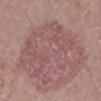biopsy status: catalogued during a skin exam; not biopsied | body site: the right lower leg | lighting: white-light illumination | lesion size: ~10.5 mm (longest diameter) | image-analysis metrics: a footprint of about 49 mm² and a symmetry-axis asymmetry near 0.45; a mean CIELAB color near L≈53 a*≈21 b*≈19, a lesion–skin lightness drop of about 7, and a lesion-to-skin contrast of about 5 (normalized; higher = more distinct); a border-irregularity rating of about 7/10, a color-variation rating of about 4/10, and radial color variation of about 1.5 | acquisition: 15 mm crop, total-body photography | subject: male, in their mid- to late 50s.